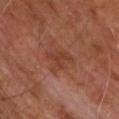| key | value |
|---|---|
| body site | the chest |
| subject | male, aged 58 to 62 |
| illumination | cross-polarized illumination |
| TBP lesion metrics | a mean CIELAB color near L≈38 a*≈25 b*≈28, roughly 7 lightness units darker than nearby skin, and a normalized lesion–skin contrast near 6 |
| lesion diameter | about 4 mm |
| image | ~15 mm crop, total-body skin-cancer survey |